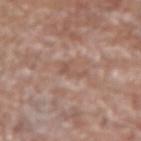Clinical impression:
This lesion was catalogued during total-body skin photography and was not selected for biopsy.
Acquisition and patient details:
The lesion is on the right forearm. A 15 mm close-up tile from a total-body photography series done for melanoma screening. A female patient, in their 80s.